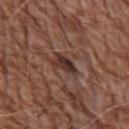Q: Was this lesion biopsied?
A: total-body-photography surveillance lesion; no biopsy
Q: What is the imaging modality?
A: total-body-photography crop, ~15 mm field of view
Q: What are the patient's age and sex?
A: male, in their 70s
Q: Lesion location?
A: the left upper arm